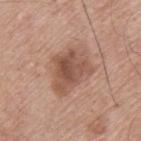notes = catalogued during a skin exam; not biopsied
lesion size = ≈5 mm
anatomic site = the upper back
image = ~15 mm crop, total-body skin-cancer survey
patient = male, approximately 75 years of age
automated lesion analysis = a mean CIELAB color near L≈52 a*≈20 b*≈28, roughly 11 lightness units darker than nearby skin, and a normalized border contrast of about 7.5; a border-irregularity rating of about 4/10, a color-variation rating of about 5/10, and a peripheral color-asymmetry measure near 1.5
lighting = white-light illumination